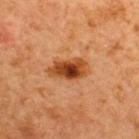tile lighting=cross-polarized illumination | diameter=~4.5 mm (longest diameter) | patient=male, aged around 65 | anatomic site=the upper back | acquisition=total-body-photography crop, ~15 mm field of view.